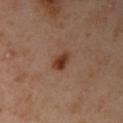The lesion was photographed on a routine skin check and not biopsied; there is no pathology result.
The lesion-visualizer software estimated a nevus-likeness score of about 100/100.
A female patient aged approximately 40.
About 3 mm across.
Located on the left arm.
A 15 mm close-up extracted from a 3D total-body photography capture.
Imaged with cross-polarized lighting.